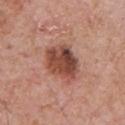<record>
<biopsy_status>not biopsied; imaged during a skin examination</biopsy_status>
<automated_metrics>
  <cielab_L>47</cielab_L>
  <cielab_a>24</cielab_a>
  <cielab_b>28</cielab_b>
  <vs_skin_contrast_norm>10.0</vs_skin_contrast_norm>
  <border_irregularity_0_10>2.0</border_irregularity_0_10>
  <color_variation_0_10>7.0</color_variation_0_10>
  <peripheral_color_asymmetry>2.5</peripheral_color_asymmetry>
</automated_metrics>
<lighting>white-light</lighting>
<lesion_size>
  <long_diameter_mm_approx>5.5</long_diameter_mm_approx>
</lesion_size>
<site>chest</site>
<image>
  <source>total-body photography crop</source>
  <field_of_view_mm>15</field_of_view_mm>
</image>
<patient>
  <sex>male</sex>
  <age_approx>70</age_approx>
</patient>
</record>